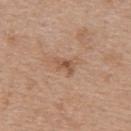- biopsy status: catalogued during a skin exam; not biopsied
- image: 15 mm crop, total-body photography
- image-analysis metrics: an area of roughly 3 mm²; a lesion–skin lightness drop of about 9 and a lesion-to-skin contrast of about 6.5 (normalized; higher = more distinct); a border-irregularity index near 3.5/10, internal color variation of about 3 on a 0–10 scale, and peripheral color asymmetry of about 1; a classifier nevus-likeness of about 0/100 and lesion-presence confidence of about 100/100
- tile lighting: white-light illumination
- subject: female, approximately 40 years of age
- site: the upper back